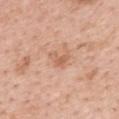Clinical impression: No biopsy was performed on this lesion — it was imaged during a full skin examination and was not determined to be concerning. Background: Longest diameter approximately 3 mm. Automated image analysis of the tile measured a lesion area of about 3.5 mm² and two-axis asymmetry of about 0.6. The analysis additionally found an average lesion color of about L≈61 a*≈22 b*≈33 (CIELAB) and a normalized lesion–skin contrast near 6. The analysis additionally found a classifier nevus-likeness of about 0/100 and lesion-presence confidence of about 100/100. A female subject, aged 48–52. Imaged with white-light lighting. A 15 mm close-up extracted from a 3D total-body photography capture. On the upper back.